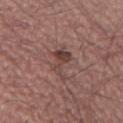<tbp_lesion>
<biopsy_status>not biopsied; imaged during a skin examination</biopsy_status>
<lesion_size>
  <long_diameter_mm_approx>4.0</long_diameter_mm_approx>
</lesion_size>
<site>leg</site>
<image>
  <source>total-body photography crop</source>
  <field_of_view_mm>15</field_of_view_mm>
</image>
<patient>
  <sex>male</sex>
  <age_approx>55</age_approx>
</patient>
<automated_metrics>
  <area_mm2_approx>6.0</area_mm2_approx>
  <eccentricity>0.85</eccentricity>
  <shape_asymmetry>0.5</shape_asymmetry>
  <cielab_L>42</cielab_L>
  <cielab_a>20</cielab_a>
  <cielab_b>21</cielab_b>
  <vs_skin_contrast_norm>7.0</vs_skin_contrast_norm>
  <border_irregularity_0_10>6.5</border_irregularity_0_10>
  <color_variation_0_10>2.5</color_variation_0_10>
  <peripheral_color_asymmetry>1.0</peripheral_color_asymmetry>
  <nevus_likeness_0_100>0</nevus_likeness_0_100>
</automated_metrics>
<lighting>white-light</lighting>
</tbp_lesion>